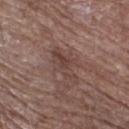Impression:
Recorded during total-body skin imaging; not selected for excision or biopsy.
Context:
A 15 mm close-up extracted from a 3D total-body photography capture. On the right thigh. A male subject approximately 70 years of age.